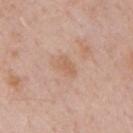Clinical impression: Part of a total-body skin-imaging series; this lesion was reviewed on a skin check and was not flagged for biopsy. Image and clinical context: Longest diameter approximately 3 mm. A male patient, aged 58–62. Captured under white-light illumination. The lesion is on the mid back. The total-body-photography lesion software estimated border irregularity of about 3 on a 0–10 scale and peripheral color asymmetry of about 0.5. A 15 mm close-up tile from a total-body photography series done for melanoma screening.